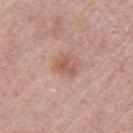The lesion was tiled from a total-body skin photograph and was not biopsied. The tile uses white-light illumination. About 3 mm across. On the left upper arm. A female patient approximately 65 years of age. A 15 mm close-up tile from a total-body photography series done for melanoma screening.